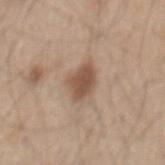Part of a total-body skin-imaging series; this lesion was reviewed on a skin check and was not flagged for biopsy. The tile uses white-light illumination. A close-up tile cropped from a whole-body skin photograph, about 15 mm across. A male subject, about 45 years old. On the back.